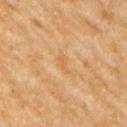body site: the right upper arm
image: 15 mm crop, total-body photography
lesion diameter: ~2.5 mm (longest diameter)
subject: female, aged 68–72